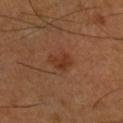The lesion was photographed on a routine skin check and not biopsied; there is no pathology result. The lesion is located on the right lower leg. The patient is a male approximately 50 years of age. A 15 mm close-up extracted from a 3D total-body photography capture.